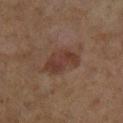Recorded during total-body skin imaging; not selected for excision or biopsy.
A 15 mm close-up extracted from a 3D total-body photography capture.
A female subject about 60 years old.
The lesion is located on the left lower leg.